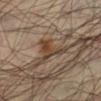patient:
  sex: male
  age_approx: 35
lesion_size:
  long_diameter_mm_approx: 4.5
site: left lower leg
lighting: cross-polarized
image:
  source: total-body photography crop
  field_of_view_mm: 15